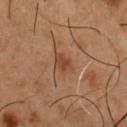<lesion>
<patient>
  <sex>male</sex>
  <age_approx>55</age_approx>
</patient>
<lighting>cross-polarized</lighting>
<image>
  <source>total-body photography crop</source>
  <field_of_view_mm>15</field_of_view_mm>
</image>
<site>chest</site>
<automated_metrics>
  <area_mm2_approx>5.0</area_mm2_approx>
  <eccentricity>0.7</eccentricity>
  <shape_asymmetry>0.25</shape_asymmetry>
</automated_metrics>
<lesion_size>
  <long_diameter_mm_approx>3.0</long_diameter_mm_approx>
</lesion_size>
</lesion>